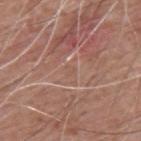{
  "biopsy_status": "not biopsied; imaged during a skin examination",
  "lesion_size": {
    "long_diameter_mm_approx": 1.5
  },
  "site": "back",
  "image": {
    "source": "total-body photography crop",
    "field_of_view_mm": 15
  },
  "patient": {
    "sex": "male",
    "age_approx": 60
  },
  "lighting": "white-light"
}